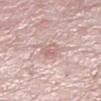Q: Was a biopsy performed?
A: no biopsy performed (imaged during a skin exam)
Q: How large is the lesion?
A: about 2.5 mm
Q: Who is the patient?
A: female, roughly 60 years of age
Q: What kind of image is this?
A: ~15 mm crop, total-body skin-cancer survey
Q: Lesion location?
A: the left lower leg
Q: How was the tile lit?
A: white-light